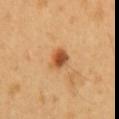Clinical impression: This lesion was catalogued during total-body skin photography and was not selected for biopsy. Background: The lesion's longest dimension is about 2.5 mm. This is a cross-polarized tile. The lesion is located on the front of the torso. The subject is a male approximately 50 years of age. The total-body-photography lesion software estimated a lesion area of about 4.5 mm², a shape eccentricity near 0.5, and a symmetry-axis asymmetry near 0.2. It also reported a lesion color around L≈52 a*≈26 b*≈40 in CIELAB, roughly 14 lightness units darker than nearby skin, and a lesion-to-skin contrast of about 9.5 (normalized; higher = more distinct). And it measured a nevus-likeness score of about 100/100 and lesion-presence confidence of about 100/100. A roughly 15 mm field-of-view crop from a total-body skin photograph.